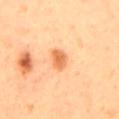{"biopsy_status": "not biopsied; imaged during a skin examination"}